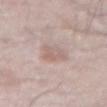<tbp_lesion>
<biopsy_status>not biopsied; imaged during a skin examination</biopsy_status>
<lighting>white-light</lighting>
<lesion_size>
  <long_diameter_mm_approx>3.0</long_diameter_mm_approx>
</lesion_size>
<site>abdomen</site>
<patient>
  <sex>male</sex>
  <age_approx>85+</age_approx>
</patient>
<image>
  <source>total-body photography crop</source>
  <field_of_view_mm>15</field_of_view_mm>
</image>
</tbp_lesion>